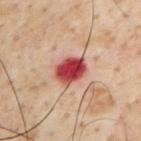Clinical impression: Part of a total-body skin-imaging series; this lesion was reviewed on a skin check and was not flagged for biopsy. Background: On the chest. The patient is a male aged approximately 55. The tile uses cross-polarized illumination. Approximately 4 mm at its widest. A 15 mm close-up tile from a total-body photography series done for melanoma screening.